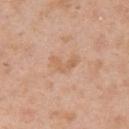Recorded during total-body skin imaging; not selected for excision or biopsy. The lesion's longest dimension is about 3 mm. From the right upper arm. The tile uses white-light illumination. A region of skin cropped from a whole-body photographic capture, roughly 15 mm wide. A female patient, aged 38–42.